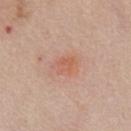{
  "biopsy_status": "not biopsied; imaged during a skin examination",
  "lighting": "white-light",
  "image": {
    "source": "total-body photography crop",
    "field_of_view_mm": 15
  },
  "patient": {
    "sex": "male",
    "age_approx": 60
  },
  "site": "chest",
  "automated_metrics": {
    "area_mm2_approx": 5.5,
    "eccentricity": 0.65,
    "shape_asymmetry": 0.2,
    "nevus_likeness_0_100": 5,
    "lesion_detection_confidence_0_100": 100
  },
  "lesion_size": {
    "long_diameter_mm_approx": 3.0
  }
}